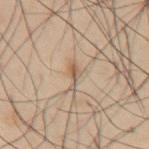<case>
  <biopsy_status>not biopsied; imaged during a skin examination</biopsy_status>
  <lighting>cross-polarized</lighting>
  <image>
    <source>total-body photography crop</source>
    <field_of_view_mm>15</field_of_view_mm>
  </image>
  <patient>
    <sex>male</sex>
    <age_approx>55</age_approx>
  </patient>
  <site>abdomen</site>
  <automated_metrics>
    <cielab_L>57</cielab_L>
    <cielab_a>15</cielab_a>
    <cielab_b>29</cielab_b>
    <vs_skin_darker_L>10.0</vs_skin_darker_L>
    <border_irregularity_0_10>5.5</border_irregularity_0_10>
    <color_variation_0_10>2.0</color_variation_0_10>
    <peripheral_color_asymmetry>0.0</peripheral_color_asymmetry>
    <nevus_likeness_0_100>0</nevus_likeness_0_100>
  </automated_metrics>
</case>